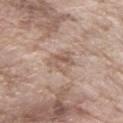Recorded during total-body skin imaging; not selected for excision or biopsy. The lesion is located on the arm. A close-up tile cropped from a whole-body skin photograph, about 15 mm across. A female patient, aged around 75.